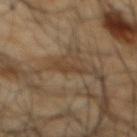Captured during whole-body skin photography for melanoma surveillance; the lesion was not biopsied.
Automated tile analysis of the lesion measured an area of roughly 3 mm², an outline eccentricity of about 0.9 (0 = round, 1 = elongated), and a symmetry-axis asymmetry near 0.4.
Approximately 3 mm at its widest.
A region of skin cropped from a whole-body photographic capture, roughly 15 mm wide.
This is a cross-polarized tile.
The subject is a male in their mid-60s.
The lesion is on the mid back.